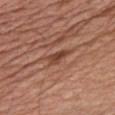Imaged during a routine full-body skin examination; the lesion was not biopsied and no histopathology is available. Automated image analysis of the tile measured a mean CIELAB color near L≈44 a*≈24 b*≈30, a lesion–skin lightness drop of about 10, and a normalized lesion–skin contrast near 8. The analysis additionally found border irregularity of about 4 on a 0–10 scale and a within-lesion color-variation index near 2/10. The tile uses white-light illumination. Cropped from a total-body skin-imaging series; the visible field is about 15 mm. About 3 mm across. A male patient, aged 68–72. On the chest.